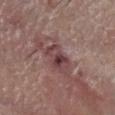Case summary:
* workup: imaged on a skin check; not biopsied
* TBP lesion metrics: a lesion-detection confidence of about 100/100
* anatomic site: the head or neck
* illumination: white-light illumination
* patient: male, roughly 80 years of age
* lesion diameter: ~3 mm (longest diameter)
* image: total-body-photography crop, ~15 mm field of view